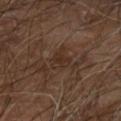No biopsy was performed on this lesion — it was imaged during a full skin examination and was not determined to be concerning.
The patient is a male aged 68–72.
On the arm.
A lesion tile, about 15 mm wide, cut from a 3D total-body photograph.